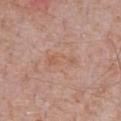<tbp_lesion>
  <lighting>white-light</lighting>
  <image>
    <source>total-body photography crop</source>
    <field_of_view_mm>15</field_of_view_mm>
  </image>
  <site>chest</site>
  <patient>
    <sex>male</sex>
    <age_approx>70</age_approx>
  </patient>
  <lesion_size>
    <long_diameter_mm_approx>4.0</long_diameter_mm_approx>
  </lesion_size>
</tbp_lesion>